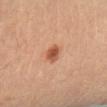<record>
<biopsy_status>not biopsied; imaged during a skin examination</biopsy_status>
<patient>
  <sex>female</sex>
  <age_approx>65</age_approx>
</patient>
<site>right lower leg</site>
<lighting>cross-polarized</lighting>
<automated_metrics>
  <border_irregularity_0_10>2.5</border_irregularity_0_10>
  <color_variation_0_10>3.5</color_variation_0_10>
  <peripheral_color_asymmetry>1.0</peripheral_color_asymmetry>
</automated_metrics>
<image>
  <source>total-body photography crop</source>
  <field_of_view_mm>15</field_of_view_mm>
</image>
</record>